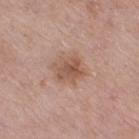{"biopsy_status": "not biopsied; imaged during a skin examination", "image": {"source": "total-body photography crop", "field_of_view_mm": 15}, "lighting": "white-light", "patient": {"sex": "female", "age_approx": 40}, "site": "right thigh", "lesion_size": {"long_diameter_mm_approx": 3.5}}